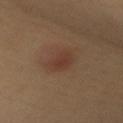Impression: Recorded during total-body skin imaging; not selected for excision or biopsy. Background: The lesion's longest dimension is about 3 mm. Cropped from a total-body skin-imaging series; the visible field is about 15 mm. Imaged with cross-polarized lighting. A female subject aged 48 to 52. The lesion is located on the back.